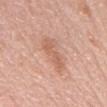| key | value |
|---|---|
| subject | male, aged around 80 |
| image-analysis metrics | a lesion color around L≈62 a*≈22 b*≈30 in CIELAB, roughly 8 lightness units darker than nearby skin, and a normalized lesion–skin contrast near 5.5; a within-lesion color-variation index near 3/10 and radial color variation of about 1 |
| lighting | white-light illumination |
| image source | ~15 mm tile from a whole-body skin photo |
| location | the chest |
| lesion diameter | ≈5.5 mm |